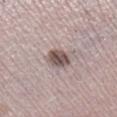The lesion was photographed on a routine skin check and not biopsied; there is no pathology result. A female subject in their 50s. A roughly 15 mm field-of-view crop from a total-body skin photograph. On the right lower leg. This is a white-light tile. The lesion's longest dimension is about 3 mm. The total-body-photography lesion software estimated a lesion area of about 6 mm² and an eccentricity of roughly 0.6. And it measured about 15 CIELAB-L* units darker than the surrounding skin and a lesion-to-skin contrast of about 10 (normalized; higher = more distinct). The analysis additionally found internal color variation of about 4.5 on a 0–10 scale and peripheral color asymmetry of about 1.5. The analysis additionally found an automated nevus-likeness rating near 80 out of 100 and a lesion-detection confidence of about 85/100.